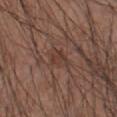Q: Was a biopsy performed?
A: catalogued during a skin exam; not biopsied
Q: How was the tile lit?
A: white-light illumination
Q: Where on the body is the lesion?
A: the right forearm
Q: What did automated image analysis measure?
A: an outline eccentricity of about 0.65 (0 = round, 1 = elongated) and two-axis asymmetry of about 0.4; a mean CIELAB color near L≈38 a*≈19 b*≈24, a lesion–skin lightness drop of about 6, and a normalized lesion–skin contrast near 5.5
Q: What is the lesion's diameter?
A: ≈2.5 mm
Q: How was this image acquired?
A: total-body-photography crop, ~15 mm field of view
Q: Who is the patient?
A: male, approximately 50 years of age